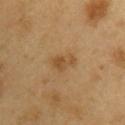{
  "biopsy_status": "not biopsied; imaged during a skin examination",
  "image": {
    "source": "total-body photography crop",
    "field_of_view_mm": 15
  },
  "lighting": "cross-polarized",
  "lesion_size": {
    "long_diameter_mm_approx": 3.0
  },
  "patient": {
    "sex": "male",
    "age_approx": 60
  },
  "automated_metrics": {
    "area_mm2_approx": 5.0,
    "eccentricity": 0.75,
    "shape_asymmetry": 0.3,
    "nevus_likeness_0_100": 30
  },
  "site": "arm"
}